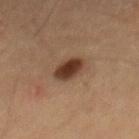Captured during whole-body skin photography for melanoma surveillance; the lesion was not biopsied. The lesion-visualizer software estimated a border-irregularity index near 1.5/10, a color-variation rating of about 3/10, and radial color variation of about 1. It also reported a nevus-likeness score of about 100/100 and a detector confidence of about 100 out of 100 that the crop contains a lesion. A 15 mm crop from a total-body photograph taken for skin-cancer surveillance. The subject is a male roughly 60 years of age. Measured at roughly 3.5 mm in maximum diameter. This is a cross-polarized tile.